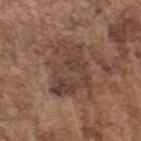Impression: Imaged during a routine full-body skin examination; the lesion was not biopsied and no histopathology is available. Clinical summary: The lesion is located on the right upper arm. A roughly 15 mm field-of-view crop from a total-body skin photograph. The subject is a male aged 73–77. Automated tile analysis of the lesion measured a lesion color around L≈41 a*≈17 b*≈24 in CIELAB, about 9 CIELAB-L* units darker than the surrounding skin, and a lesion-to-skin contrast of about 7.5 (normalized; higher = more distinct). The tile uses white-light illumination.